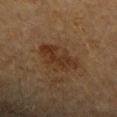<tbp_lesion>
  <biopsy_status>not biopsied; imaged during a skin examination</biopsy_status>
  <site>arm</site>
  <lesion_size>
    <long_diameter_mm_approx>5.5</long_diameter_mm_approx>
  </lesion_size>
  <image>
    <source>total-body photography crop</source>
    <field_of_view_mm>15</field_of_view_mm>
  </image>
  <patient>
    <sex>female</sex>
    <age_approx>60</age_approx>
  </patient>
  <automated_metrics>
    <area_mm2_approx>12.0</area_mm2_approx>
    <eccentricity>0.8</eccentricity>
    <shape_asymmetry>0.3</shape_asymmetry>
    <color_variation_0_10>3.5</color_variation_0_10>
    <peripheral_color_asymmetry>1.0</peripheral_color_asymmetry>
  </automated_metrics>
  <lighting>cross-polarized</lighting>
</tbp_lesion>